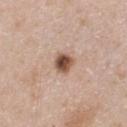notes — no biopsy performed (imaged during a skin exam)
acquisition — total-body-photography crop, ~15 mm field of view
subject — male, aged approximately 40
automated lesion analysis — an automated nevus-likeness rating near 100 out of 100
lesion diameter — ≈2.5 mm
anatomic site — the upper back
illumination — white-light